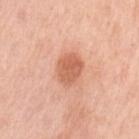Captured during whole-body skin photography for melanoma surveillance; the lesion was not biopsied. Located on the left upper arm. Captured under white-light illumination. Approximately 3.5 mm at its widest. A 15 mm close-up extracted from a 3D total-body photography capture. The patient is a female aged 48 to 52. An algorithmic analysis of the crop reported about 11 CIELAB-L* units darker than the surrounding skin and a lesion-to-skin contrast of about 7 (normalized; higher = more distinct).